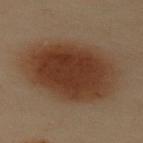Assessment:
The lesion was tiled from a total-body skin photograph and was not biopsied.
Image and clinical context:
The lesion is on the back. A female subject, aged 58 to 62. A 15 mm close-up extracted from a 3D total-body photography capture.